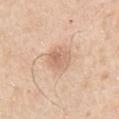Notes:
- patient: male, approximately 55 years of age
- tile lighting: white-light
- image-analysis metrics: a footprint of about 5.5 mm² and an eccentricity of roughly 0.5; a mean CIELAB color near L≈65 a*≈20 b*≈31, a lesion–skin lightness drop of about 9, and a normalized border contrast of about 5.5; a classifier nevus-likeness of about 60/100 and lesion-presence confidence of about 100/100
- anatomic site: the right upper arm
- lesion size: ~3 mm (longest diameter)
- acquisition: total-body-photography crop, ~15 mm field of view